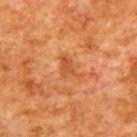Clinical summary: Automated image analysis of the tile measured an average lesion color of about L≈41 a*≈24 b*≈36 (CIELAB) and about 7 CIELAB-L* units darker than the surrounding skin. This is a cross-polarized tile. Measured at roughly 3.5 mm in maximum diameter. Located on the upper back. A 15 mm close-up extracted from a 3D total-body photography capture. The patient is a male aged 78 to 82.